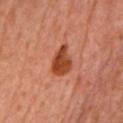<lesion>
<biopsy_status>not biopsied; imaged during a skin examination</biopsy_status>
<site>chest</site>
<image>
  <source>total-body photography crop</source>
  <field_of_view_mm>15</field_of_view_mm>
</image>
<patient>
  <sex>female</sex>
  <age_approx>60</age_approx>
</patient>
<lesion_size>
  <long_diameter_mm_approx>4.0</long_diameter_mm_approx>
</lesion_size>
<automated_metrics>
  <area_mm2_approx>7.5</area_mm2_approx>
  <eccentricity>0.8</eccentricity>
  <shape_asymmetry>0.25</shape_asymmetry>
  <cielab_L>47</cielab_L>
  <cielab_a>31</cielab_a>
  <cielab_b>39</cielab_b>
  <vs_skin_darker_L>14.0</vs_skin_darker_L>
  <vs_skin_contrast_norm>10.5</vs_skin_contrast_norm>
  <nevus_likeness_0_100>100</nevus_likeness_0_100>
  <lesion_detection_confidence_0_100>100</lesion_detection_confidence_0_100>
</automated_metrics>
</lesion>